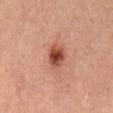<record>
<biopsy_status>not biopsied; imaged during a skin examination</biopsy_status>
<image>
  <source>total-body photography crop</source>
  <field_of_view_mm>15</field_of_view_mm>
</image>
<automated_metrics>
  <area_mm2_approx>7.0</area_mm2_approx>
  <shape_asymmetry>0.2</shape_asymmetry>
  <nevus_likeness_0_100>95</nevus_likeness_0_100>
  <lesion_detection_confidence_0_100>100</lesion_detection_confidence_0_100>
</automated_metrics>
<site>mid back</site>
<lighting>cross-polarized</lighting>
<lesion_size>
  <long_diameter_mm_approx>3.5</long_diameter_mm_approx>
</lesion_size>
<patient>
  <sex>male</sex>
  <age_approx>50</age_approx>
</patient>
</record>